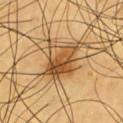<case>
  <lighting>cross-polarized</lighting>
  <automated_metrics>
    <border_irregularity_0_10>3.5</border_irregularity_0_10>
    <color_variation_0_10>9.0</color_variation_0_10>
  </automated_metrics>
  <patient>
    <sex>male</sex>
    <age_approx>60</age_approx>
  </patient>
  <image>
    <source>total-body photography crop</source>
    <field_of_view_mm>15</field_of_view_mm>
  </image>
  <site>chest</site>
  <lesion_size>
    <long_diameter_mm_approx>5.0</long_diameter_mm_approx>
  </lesion_size>
</case>